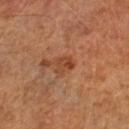Q: Was this lesion biopsied?
A: catalogued during a skin exam; not biopsied
Q: How large is the lesion?
A: about 2.5 mm
Q: What is the imaging modality?
A: 15 mm crop, total-body photography
Q: What lighting was used for the tile?
A: cross-polarized
Q: What are the patient's age and sex?
A: male, roughly 65 years of age